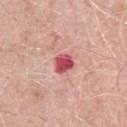{
  "biopsy_status": "not biopsied; imaged during a skin examination",
  "lighting": "white-light",
  "image": {
    "source": "total-body photography crop",
    "field_of_view_mm": 15
  },
  "patient": {
    "sex": "male",
    "age_approx": 70
  },
  "site": "chest"
}